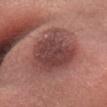Imaged during a routine full-body skin examination; the lesion was not biopsied and no histopathology is available. A lesion tile, about 15 mm wide, cut from a 3D total-body photograph. Captured under white-light illumination. Approximately 6 mm at its widest. The lesion-visualizer software estimated an average lesion color of about L≈43 a*≈23 b*≈22 (CIELAB) and roughly 12 lightness units darker than nearby skin. The analysis additionally found radial color variation of about 1.5. On the head or neck. A female subject, about 65 years old.